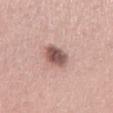This lesion was catalogued during total-body skin photography and was not selected for biopsy.
The recorded lesion diameter is about 4 mm.
Imaged with white-light lighting.
On the left thigh.
A 15 mm close-up tile from a total-body photography series done for melanoma screening.
A male subject in their 50s.